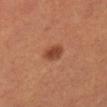Assessment: This lesion was catalogued during total-body skin photography and was not selected for biopsy. Context: A 15 mm close-up extracted from a 3D total-body photography capture. A male patient in their 50s. Located on the leg. Automated tile analysis of the lesion measured an average lesion color of about L≈44 a*≈26 b*≈33 (CIELAB), a lesion–skin lightness drop of about 11, and a lesion-to-skin contrast of about 8.5 (normalized; higher = more distinct). The software also gave a classifier nevus-likeness of about 100/100 and lesion-presence confidence of about 100/100. The tile uses cross-polarized illumination.